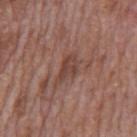workup = imaged on a skin check; not biopsied
imaging modality = 15 mm crop, total-body photography
body site = the mid back
patient = male, about 70 years old